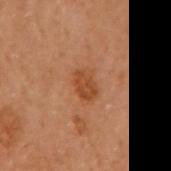{"lighting": "cross-polarized", "image": {"source": "total-body photography crop", "field_of_view_mm": 15}, "lesion_size": {"long_diameter_mm_approx": 3.0}, "site": "right arm", "patient": {"sex": "female", "age_approx": 60}}